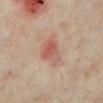Case summary:
- follow-up: no biopsy performed (imaged during a skin exam)
- subject: female, aged approximately 35
- image: ~15 mm crop, total-body skin-cancer survey
- location: the left lower leg